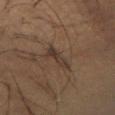<lesion>
<biopsy_status>not biopsied; imaged during a skin examination</biopsy_status>
</lesion>